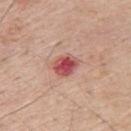Assessment: No biopsy was performed on this lesion — it was imaged during a full skin examination and was not determined to be concerning. Context: The lesion is located on the back. A lesion tile, about 15 mm wide, cut from a 3D total-body photograph. The total-body-photography lesion software estimated a lesion area of about 6 mm², an eccentricity of roughly 0.6, and a shape-asymmetry score of about 0.2 (0 = symmetric). The analysis additionally found a nevus-likeness score of about 0/100 and lesion-presence confidence of about 100/100. The tile uses white-light illumination. A male patient in their mid-50s. Approximately 3 mm at its widest.